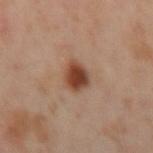follow-up = imaged on a skin check; not biopsied | body site = the left arm | image source = 15 mm crop, total-body photography | lighting = cross-polarized illumination | lesion diameter = about 3 mm | subject = female, aged around 40.